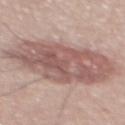This lesion was catalogued during total-body skin photography and was not selected for biopsy. Cropped from a whole-body photographic skin survey; the tile spans about 15 mm. Imaged with white-light lighting. The lesion is on the mid back. The recorded lesion diameter is about 10.5 mm. Automated tile analysis of the lesion measured an area of roughly 47 mm² and an eccentricity of roughly 0.8. The software also gave border irregularity of about 2 on a 0–10 scale, internal color variation of about 5 on a 0–10 scale, and radial color variation of about 2. A male subject aged 53 to 57.